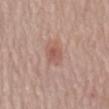This lesion was catalogued during total-body skin photography and was not selected for biopsy. A 15 mm crop from a total-body photograph taken for skin-cancer surveillance. The lesion's longest dimension is about 3 mm. From the mid back. A female subject, approximately 65 years of age. The tile uses white-light illumination.